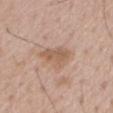Captured during whole-body skin photography for melanoma surveillance; the lesion was not biopsied.